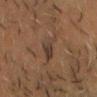Notes:
- workup: total-body-photography surveillance lesion; no biopsy
- lighting: cross-polarized
- patient: male, in their 60s
- image: ~15 mm crop, total-body skin-cancer survey
- automated metrics: an area of roughly 5.5 mm²; border irregularity of about 4.5 on a 0–10 scale, internal color variation of about 2.5 on a 0–10 scale, and radial color variation of about 1
- diameter: about 3.5 mm
- body site: the head or neck